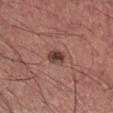Assessment:
The lesion was photographed on a routine skin check and not biopsied; there is no pathology result.
Acquisition and patient details:
The recorded lesion diameter is about 2.5 mm. Imaged with white-light lighting. The lesion is located on the right lower leg. The subject is a male in their 40s. A roughly 15 mm field-of-view crop from a total-body skin photograph.